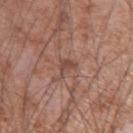Q: What is the anatomic site?
A: the left upper arm
Q: What is the lesion's diameter?
A: about 2.5 mm
Q: What kind of image is this?
A: ~15 mm tile from a whole-body skin photo
Q: What did automated image analysis measure?
A: a lesion area of about 3.5 mm², an eccentricity of roughly 0.7, and a symmetry-axis asymmetry near 0.4; an average lesion color of about L≈47 a*≈20 b*≈25 (CIELAB), roughly 8 lightness units darker than nearby skin, and a lesion-to-skin contrast of about 6 (normalized; higher = more distinct); a classifier nevus-likeness of about 0/100 and a lesion-detection confidence of about 75/100
Q: Patient demographics?
A: male, about 75 years old
Q: What lighting was used for the tile?
A: white-light